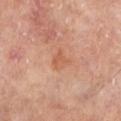The lesion was photographed on a routine skin check and not biopsied; there is no pathology result. This image is a 15 mm lesion crop taken from a total-body photograph. A male subject about 65 years old. Located on the left lower leg.